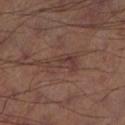The lesion was tiled from a total-body skin photograph and was not biopsied. The recorded lesion diameter is about 5 mm. The total-body-photography lesion software estimated a border-irregularity index near 6.5/10 and a within-lesion color-variation index near 3.5/10. The analysis additionally found an automated nevus-likeness rating near 0 out of 100 and a detector confidence of about 95 out of 100 that the crop contains a lesion. A lesion tile, about 15 mm wide, cut from a 3D total-body photograph. Captured under cross-polarized illumination. From the left lower leg.